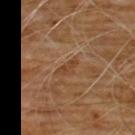Recorded during total-body skin imaging; not selected for excision or biopsy. The patient is a male roughly 60 years of age. The tile uses cross-polarized illumination. A region of skin cropped from a whole-body photographic capture, roughly 15 mm wide. The lesion is on the chest.